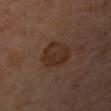follow-up: catalogued during a skin exam; not biopsied
location: the left forearm
image: ~15 mm tile from a whole-body skin photo
subject: female, in their mid- to late 50s
size: ≈4 mm